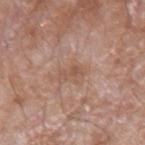Assessment: The lesion was photographed on a routine skin check and not biopsied; there is no pathology result. Context: Captured under white-light illumination. Measured at roughly 2.5 mm in maximum diameter. A male patient aged 58 to 62. Automated image analysis of the tile measured a border-irregularity index near 4.5/10, internal color variation of about 0.5 on a 0–10 scale, and radial color variation of about 0. And it measured lesion-presence confidence of about 100/100. Cropped from a whole-body photographic skin survey; the tile spans about 15 mm. The lesion is located on the arm.